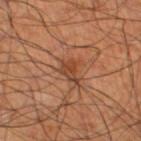Impression:
This lesion was catalogued during total-body skin photography and was not selected for biopsy.
Background:
About 3 mm across. Imaged with cross-polarized lighting. The lesion is on the left thigh. A male patient roughly 60 years of age. A 15 mm close-up extracted from a 3D total-body photography capture.